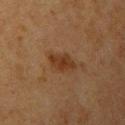workup: no biopsy performed (imaged during a skin exam) | patient: female, aged around 55 | site: the left upper arm | image: total-body-photography crop, ~15 mm field of view.